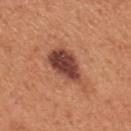Case summary:
• biopsy status — catalogued during a skin exam; not biopsied
• body site — the mid back
• subject — male, about 55 years old
• size — ~6 mm (longest diameter)
• lighting — white-light
• image — total-body-photography crop, ~15 mm field of view
• TBP lesion metrics — an area of roughly 13 mm² and a symmetry-axis asymmetry near 0.3; a lesion color around L≈45 a*≈25 b*≈29 in CIELAB, a lesion–skin lightness drop of about 15, and a normalized border contrast of about 11.5; border irregularity of about 4 on a 0–10 scale, internal color variation of about 5 on a 0–10 scale, and peripheral color asymmetry of about 1.5